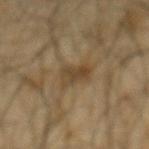imaging modality = ~15 mm crop, total-body skin-cancer survey
subject = male, about 65 years old
illumination = cross-polarized
TBP lesion metrics = a lesion area of about 5.5 mm²; a mean CIELAB color near L≈40 a*≈12 b*≈30, a lesion–skin lightness drop of about 8, and a normalized border contrast of about 7; a border-irregularity rating of about 3.5/10, internal color variation of about 3.5 on a 0–10 scale, and a peripheral color-asymmetry measure near 1; an automated nevus-likeness rating near 10 out of 100
size = ~3 mm (longest diameter)
body site = the mid back